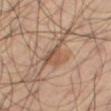Clinical impression:
Imaged during a routine full-body skin examination; the lesion was not biopsied and no histopathology is available.
Acquisition and patient details:
This image is a 15 mm lesion crop taken from a total-body photograph. The recorded lesion diameter is about 5 mm. Captured under cross-polarized illumination. A male patient approximately 60 years of age. The lesion is on the right thigh.